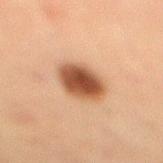biopsy status = imaged on a skin check; not biopsied
acquisition = total-body-photography crop, ~15 mm field of view
lesion diameter = ~4 mm (longest diameter)
patient = female, aged approximately 55
body site = the right lower leg